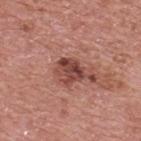Assessment:
Captured during whole-body skin photography for melanoma surveillance; the lesion was not biopsied.
Background:
A male subject about 70 years old. Imaged with white-light lighting. From the upper back. Cropped from a total-body skin-imaging series; the visible field is about 15 mm. Automated tile analysis of the lesion measured an automated nevus-likeness rating near 30 out of 100 and lesion-presence confidence of about 100/100. Approximately 3 mm at its widest.